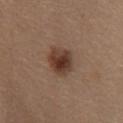Q: What did automated image analysis measure?
A: an area of roughly 9.5 mm², an eccentricity of roughly 0.65, and a symmetry-axis asymmetry near 0.15; a border-irregularity rating of about 1.5/10, a color-variation rating of about 5.5/10, and peripheral color asymmetry of about 1.5
Q: How was this image acquired?
A: ~15 mm tile from a whole-body skin photo
Q: What is the anatomic site?
A: the front of the torso
Q: What is the lesion's diameter?
A: about 4 mm
Q: Who is the patient?
A: female, aged around 65
Q: Illumination type?
A: white-light illumination